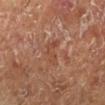<case>
<biopsy_status>not biopsied; imaged during a skin examination</biopsy_status>
<image>
  <source>total-body photography crop</source>
  <field_of_view_mm>15</field_of_view_mm>
</image>
<site>left lower leg</site>
<lighting>cross-polarized</lighting>
<patient>
  <sex>male</sex>
  <age_approx>75</age_approx>
</patient>
</case>